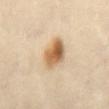Imaged during a routine full-body skin examination; the lesion was not biopsied and no histopathology is available. Captured under cross-polarized illumination. This image is a 15 mm lesion crop taken from a total-body photograph. About 4 mm across. The lesion is on the abdomen. A female patient aged approximately 50. Automated image analysis of the tile measured a footprint of about 10 mm², an eccentricity of roughly 0.75, and a shape-asymmetry score of about 0.2 (0 = symmetric).